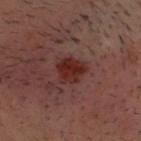Acquisition and patient details:
A male subject aged around 35. This image is a 15 mm lesion crop taken from a total-body photograph. Approximately 3.5 mm at its widest. The lesion is on the head or neck. The lesion-visualizer software estimated an average lesion color of about L≈27 a*≈24 b*≈22 (CIELAB), roughly 10 lightness units darker than nearby skin, and a normalized border contrast of about 10.
Conclusion:
On excision, pathology confirmed an intradermal melanocytic nevus — a benign skin lesion.